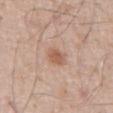The lesion was photographed on a routine skin check and not biopsied; there is no pathology result.
A roughly 15 mm field-of-view crop from a total-body skin photograph.
Automated image analysis of the tile measured a lesion area of about 4.5 mm² and a shape-asymmetry score of about 0.15 (0 = symmetric). The software also gave a mean CIELAB color near L≈58 a*≈20 b*≈30 and a lesion–skin lightness drop of about 9.
The lesion is located on the abdomen.
A male subject in their mid- to late 60s.
The tile uses white-light illumination.
Measured at roughly 2.5 mm in maximum diameter.